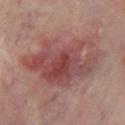follow-up = no biopsy performed (imaged during a skin exam) | body site = the left lower leg | image = ~15 mm crop, total-body skin-cancer survey | automated metrics = a footprint of about 38 mm² and a symmetry-axis asymmetry near 0.3; a border-irregularity rating of about 5.5/10 and a within-lesion color-variation index near 8/10 | patient = male, aged 68 to 72 | lighting = cross-polarized.